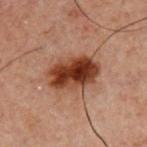This lesion was catalogued during total-body skin photography and was not selected for biopsy. A male patient, about 60 years old. From the chest. Captured under cross-polarized illumination. A lesion tile, about 15 mm wide, cut from a 3D total-body photograph. Approximately 5.5 mm at its widest.